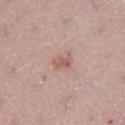Q: How was the tile lit?
A: white-light illumination
Q: What is the anatomic site?
A: the left lower leg
Q: How large is the lesion?
A: ≈2.5 mm
Q: What is the imaging modality?
A: ~15 mm tile from a whole-body skin photo
Q: Who is the patient?
A: female, roughly 40 years of age